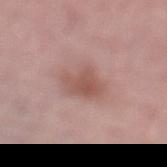* biopsy status — imaged on a skin check; not biopsied
* automated metrics — a lesion area of about 8.5 mm² and an outline eccentricity of about 0.8 (0 = round, 1 = elongated); a mean CIELAB color near L≈54 a*≈22 b*≈24, a lesion–skin lightness drop of about 9, and a normalized lesion–skin contrast near 7; border irregularity of about 3 on a 0–10 scale, a within-lesion color-variation index near 3/10, and peripheral color asymmetry of about 1
* tile lighting — white-light illumination
* lesion diameter — ~4 mm (longest diameter)
* body site — the left lower leg
* subject — female, in their mid-60s
* imaging modality — 15 mm crop, total-body photography